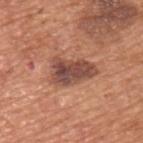– notes: total-body-photography surveillance lesion; no biopsy
– patient: male, aged 63–67
– lighting: white-light illumination
– location: the left upper arm
– lesion diameter: about 5.5 mm
– automated lesion analysis: a lesion–skin lightness drop of about 13 and a normalized border contrast of about 10; border irregularity of about 4 on a 0–10 scale and a within-lesion color-variation index near 5/10; an automated nevus-likeness rating near 5 out of 100
– acquisition: ~15 mm tile from a whole-body skin photo